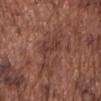Q: Was a biopsy performed?
A: no biopsy performed (imaged during a skin exam)
Q: Lesion location?
A: the front of the torso
Q: What is the lesion's diameter?
A: ≈7.5 mm
Q: How was the tile lit?
A: white-light illumination
Q: How was this image acquired?
A: 15 mm crop, total-body photography
Q: What are the patient's age and sex?
A: male, aged around 75